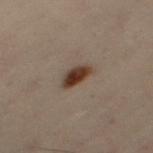Captured during whole-body skin photography for melanoma surveillance; the lesion was not biopsied. Cropped from a total-body skin-imaging series; the visible field is about 15 mm. The subject is a female aged 48 to 52. The lesion is located on the left thigh. The tile uses cross-polarized illumination.